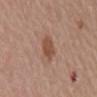Captured during whole-body skin photography for melanoma surveillance; the lesion was not biopsied.
A female subject aged 68–72.
The lesion is on the mid back.
The lesion's longest dimension is about 3 mm.
A roughly 15 mm field-of-view crop from a total-body skin photograph.
The tile uses white-light illumination.